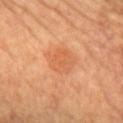location: the chest
lighting: cross-polarized illumination
lesion diameter: about 3.5 mm
patient: female, about 65 years old
image source: 15 mm crop, total-body photography